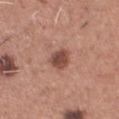follow-up: imaged on a skin check; not biopsied | subject: male, roughly 45 years of age | acquisition: ~15 mm tile from a whole-body skin photo | body site: the chest | tile lighting: white-light illumination.